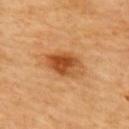Acquisition and patient details:
Measured at roughly 4.5 mm in maximum diameter. On the upper back. A female subject aged around 60. Automated tile analysis of the lesion measured a lesion area of about 11 mm². A close-up tile cropped from a whole-body skin photograph, about 15 mm across.